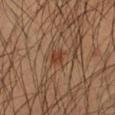The lesion was tiled from a total-body skin photograph and was not biopsied. A male patient, in their mid-40s. Longest diameter approximately 2 mm. An algorithmic analysis of the crop reported a footprint of about 3 mm², an eccentricity of roughly 0.65, and a symmetry-axis asymmetry near 0.2. The analysis additionally found a classifier nevus-likeness of about 80/100. Imaged with cross-polarized lighting. On the left forearm. A 15 mm close-up tile from a total-body photography series done for melanoma screening.